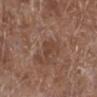biopsy_status: not biopsied; imaged during a skin examination
patient:
  sex: female
  age_approx: 80
site: leg
image:
  source: total-body photography crop
  field_of_view_mm: 15
automated_metrics:
  vs_skin_darker_L: 6.0
  vs_skin_contrast_norm: 5.0
  nevus_likeness_0_100: 0
  lesion_detection_confidence_0_100: 100
lighting: white-light